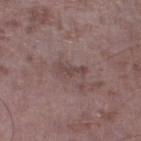biopsy_status: not biopsied; imaged during a skin examination
site: left lower leg
lesion_size:
  long_diameter_mm_approx: 3.0
image:
  source: total-body photography crop
  field_of_view_mm: 15
lighting: white-light
patient:
  sex: male
  age_approx: 50